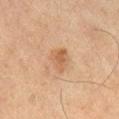workup=no biopsy performed (imaged during a skin exam)
anatomic site=the right lower leg
acquisition=total-body-photography crop, ~15 mm field of view
subject=male, aged approximately 65
size=~3.5 mm (longest diameter)